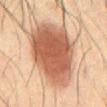Impression:
Captured during whole-body skin photography for melanoma surveillance; the lesion was not biopsied.
Context:
The patient is a male in their 60s. A 15 mm close-up extracted from a 3D total-body photography capture. From the abdomen.